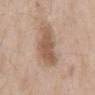The lesion was tiled from a total-body skin photograph and was not biopsied. About 5.5 mm across. On the mid back. The subject is a male aged around 65. Cropped from a whole-body photographic skin survey; the tile spans about 15 mm. The tile uses white-light illumination.